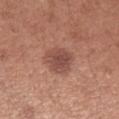  automated_metrics:
    nevus_likeness_0_100: 50
    lesion_detection_confidence_0_100: 100
  lighting: white-light
  site: right forearm
  lesion_size:
    long_diameter_mm_approx: 4.0
  image:
    source: total-body photography crop
    field_of_view_mm: 15
  patient:
    sex: female
    age_approx: 30